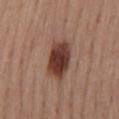Q: What is the anatomic site?
A: the mid back
Q: Lesion size?
A: ~5 mm (longest diameter)
Q: Who is the patient?
A: female, about 55 years old
Q: What kind of image is this?
A: 15 mm crop, total-body photography
Q: Illumination type?
A: white-light illumination